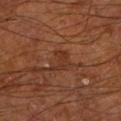workup: total-body-photography surveillance lesion; no biopsy | size: ~3 mm (longest diameter) | location: the left lower leg | automated lesion analysis: an area of roughly 3 mm², a shape eccentricity near 0.85, and a shape-asymmetry score of about 0.55 (0 = symmetric); a classifier nevus-likeness of about 0/100 and a lesion-detection confidence of about 100/100 | tile lighting: cross-polarized illumination | subject: male, aged around 65 | acquisition: ~15 mm crop, total-body skin-cancer survey.